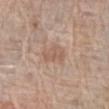Q: What are the patient's age and sex?
A: female, about 65 years old
Q: How was this image acquired?
A: total-body-photography crop, ~15 mm field of view
Q: What is the anatomic site?
A: the left arm